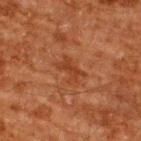Part of a total-body skin-imaging series; this lesion was reviewed on a skin check and was not flagged for biopsy. Located on the upper back. A close-up tile cropped from a whole-body skin photograph, about 15 mm across. A male patient aged around 60.